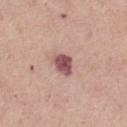Q: Is there a histopathology result?
A: imaged on a skin check; not biopsied
Q: Who is the patient?
A: female, in their mid-60s
Q: Lesion location?
A: the left thigh
Q: What is the imaging modality?
A: ~15 mm crop, total-body skin-cancer survey